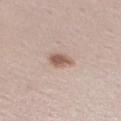This lesion was catalogued during total-body skin photography and was not selected for biopsy. A female subject roughly 40 years of age. Longest diameter approximately 3 mm. Captured under white-light illumination. Cropped from a total-body skin-imaging series; the visible field is about 15 mm. The lesion is located on the right lower leg.